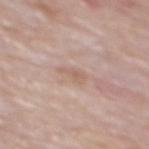Q: Was this lesion biopsied?
A: catalogued during a skin exam; not biopsied
Q: What are the patient's age and sex?
A: male, aged approximately 75
Q: What is the anatomic site?
A: the mid back
Q: Illumination type?
A: white-light illumination
Q: Lesion size?
A: ~2.5 mm (longest diameter)
Q: What kind of image is this?
A: ~15 mm crop, total-body skin-cancer survey